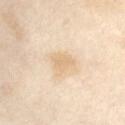No biopsy was performed on this lesion — it was imaged during a full skin examination and was not determined to be concerning.
A 15 mm crop from a total-body photograph taken for skin-cancer surveillance.
An algorithmic analysis of the crop reported a lesion area of about 5.5 mm², a shape eccentricity near 0.5, and a shape-asymmetry score of about 0.4 (0 = symmetric). The software also gave a nevus-likeness score of about 0/100 and a lesion-detection confidence of about 100/100.
Located on the abdomen.
Captured under cross-polarized illumination.
A female subject, approximately 55 years of age.